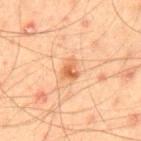Case summary:
* follow-up: no biopsy performed (imaged during a skin exam)
* patient: male, in their mid- to late 40s
* body site: the mid back
* image source: total-body-photography crop, ~15 mm field of view